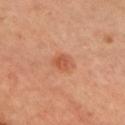follow-up — total-body-photography surveillance lesion; no biopsy
patient — female, approximately 45 years of age
anatomic site — the chest
diameter — about 2.5 mm
illumination — cross-polarized illumination
image — ~15 mm tile from a whole-body skin photo